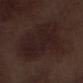Assessment:
Part of a total-body skin-imaging series; this lesion was reviewed on a skin check and was not flagged for biopsy.
Clinical summary:
A lesion tile, about 15 mm wide, cut from a 3D total-body photograph. The lesion is on the left lower leg. Measured at roughly 8 mm in maximum diameter. Imaged with white-light lighting. An algorithmic analysis of the crop reported a lesion area of about 42 mm², a shape eccentricity near 0.6, and a symmetry-axis asymmetry near 0.3. The analysis additionally found a classifier nevus-likeness of about 0/100 and a lesion-detection confidence of about 100/100. The subject is a male aged approximately 70.